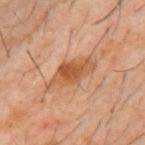Acquisition and patient details:
The total-body-photography lesion software estimated a footprint of about 11 mm², an eccentricity of roughly 0.9, and a shape-asymmetry score of about 0.25 (0 = symmetric). The software also gave a lesion color around L≈51 a*≈22 b*≈35 in CIELAB and a lesion-to-skin contrast of about 7.5 (normalized; higher = more distinct). The lesion's longest dimension is about 6 mm. Located on the mid back. A male patient in their 60s. A roughly 15 mm field-of-view crop from a total-body skin photograph.